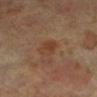- biopsy status · catalogued during a skin exam; not biopsied
- imaging modality · 15 mm crop, total-body photography
- patient · female, approximately 65 years of age
- location · the left leg